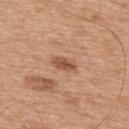follow-up=imaged on a skin check; not biopsied
patient=male, about 65 years old
anatomic site=the back
imaging modality=~15 mm tile from a whole-body skin photo